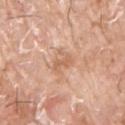Captured during whole-body skin photography for melanoma surveillance; the lesion was not biopsied. The total-body-photography lesion software estimated an area of roughly 3 mm², a shape eccentricity near 0.85, and a shape-asymmetry score of about 0.3 (0 = symmetric). The software also gave a border-irregularity index near 3.5/10, a within-lesion color-variation index near 0/10, and a peripheral color-asymmetry measure near 0. The software also gave a detector confidence of about 100 out of 100 that the crop contains a lesion. The lesion is on the front of the torso. A male subject aged 73–77. Captured under white-light illumination. A 15 mm close-up extracted from a 3D total-body photography capture. Measured at roughly 2.5 mm in maximum diameter.